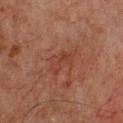This lesion was catalogued during total-body skin photography and was not selected for biopsy.
A 15 mm close-up extracted from a 3D total-body photography capture.
The lesion is on the chest.
A male patient, approximately 60 years of age.